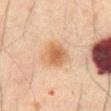Captured during whole-body skin photography for melanoma surveillance; the lesion was not biopsied. A male subject, about 70 years old. Imaged with cross-polarized lighting. A roughly 15 mm field-of-view crop from a total-body skin photograph. The lesion is on the mid back.